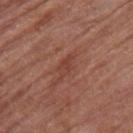| field | value |
|---|---|
| notes | catalogued during a skin exam; not biopsied |
| patient | female, aged approximately 80 |
| location | the right thigh |
| acquisition | ~15 mm tile from a whole-body skin photo |
| illumination | white-light |
| lesion diameter | about 3 mm |